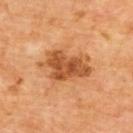- notes: imaged on a skin check; not biopsied
- location: the upper back
- lesion size: about 6.5 mm
- imaging modality: ~15 mm tile from a whole-body skin photo
- patient: male, aged 58 to 62
- lighting: cross-polarized illumination